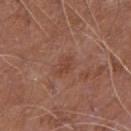follow-up = no biopsy performed (imaged during a skin exam); image = 15 mm crop, total-body photography; location = the right upper arm; lesion diameter = ≈2.5 mm; patient = male, approximately 65 years of age; lighting = white-light.